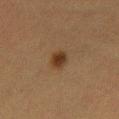The lesion was photographed on a routine skin check and not biopsied; there is no pathology result. A 15 mm close-up tile from a total-body photography series done for melanoma screening. The lesion's longest dimension is about 2.5 mm. The lesion is located on the left thigh. A female subject, in their mid-50s. This is a cross-polarized tile.